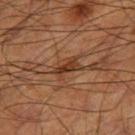Impression: Captured during whole-body skin photography for melanoma surveillance; the lesion was not biopsied. Image and clinical context: From the right thigh. Captured under cross-polarized illumination. A 15 mm close-up extracted from a 3D total-body photography capture. A male subject, aged around 60. About 3.5 mm across. The total-body-photography lesion software estimated a footprint of about 5.5 mm², an eccentricity of roughly 0.8, and two-axis asymmetry of about 0.35. And it measured a mean CIELAB color near L≈37 a*≈22 b*≈31, a lesion–skin lightness drop of about 10, and a normalized lesion–skin contrast near 8.5. The analysis additionally found a border-irregularity rating of about 4/10, a color-variation rating of about 3.5/10, and radial color variation of about 1.